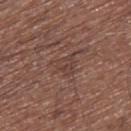| feature | finding |
|---|---|
| follow-up | catalogued during a skin exam; not biopsied |
| imaging modality | 15 mm crop, total-body photography |
| lesion size | ≈3.5 mm |
| tile lighting | white-light illumination |
| subject | male, aged approximately 75 |
| automated metrics | a shape eccentricity near 0.45 and two-axis asymmetry of about 0.45; an average lesion color of about L≈41 a*≈18 b*≈23 (CIELAB), about 6 CIELAB-L* units darker than the surrounding skin, and a lesion-to-skin contrast of about 5 (normalized; higher = more distinct); a border-irregularity index near 5/10, a color-variation rating of about 3/10, and radial color variation of about 1 |
| anatomic site | the right thigh |